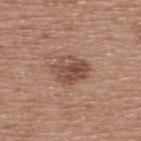follow-up: catalogued during a skin exam; not biopsied | acquisition: 15 mm crop, total-body photography | location: the upper back | image-analysis metrics: a footprint of about 9.5 mm² and a shape-asymmetry score of about 0.2 (0 = symmetric); border irregularity of about 2.5 on a 0–10 scale and radial color variation of about 2; a nevus-likeness score of about 80/100 and a lesion-detection confidence of about 100/100 | size: ≈4 mm | subject: male, aged around 65.